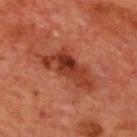<tbp_lesion>
<patient>
  <sex>male</sex>
  <age_approx>70</age_approx>
</patient>
<image>
  <source>total-body photography crop</source>
  <field_of_view_mm>15</field_of_view_mm>
</image>
<lighting>cross-polarized</lighting>
<lesion_size>
  <long_diameter_mm_approx>8.0</long_diameter_mm_approx>
</lesion_size>
<site>upper back</site>
</tbp_lesion>